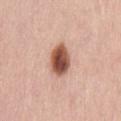Captured during whole-body skin photography for melanoma surveillance; the lesion was not biopsied.
From the back.
A female subject approximately 45 years of age.
This image is a 15 mm lesion crop taken from a total-body photograph.